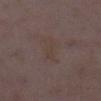The lesion was photographed on a routine skin check and not biopsied; there is no pathology result.
On the leg.
A female patient aged 28 to 32.
The lesion-visualizer software estimated an area of roughly 6.5 mm², a shape eccentricity near 0.85, and a symmetry-axis asymmetry near 0.25. The software also gave a detector confidence of about 100 out of 100 that the crop contains a lesion.
Captured under white-light illumination.
A close-up tile cropped from a whole-body skin photograph, about 15 mm across.
About 4 mm across.